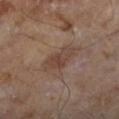Case summary:
- workup — no biopsy performed (imaged during a skin exam)
- diameter — about 4 mm
- imaging modality — total-body-photography crop, ~15 mm field of view
- anatomic site — the left lower leg
- patient — male, about 65 years old
- lighting — cross-polarized